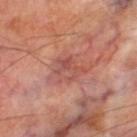Recorded during total-body skin imaging; not selected for excision or biopsy. This is a cross-polarized tile. Automated tile analysis of the lesion measured a shape eccentricity near 0.65 and a shape-asymmetry score of about 0.4 (0 = symmetric). The software also gave roughly 7 lightness units darker than nearby skin. The analysis additionally found a classifier nevus-likeness of about 0/100 and a detector confidence of about 95 out of 100 that the crop contains a lesion. A close-up tile cropped from a whole-body skin photograph, about 15 mm across. A male subject aged 68 to 72. Located on the left thigh.